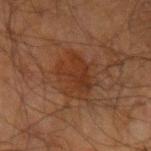<lesion>
<biopsy_status>not biopsied; imaged during a skin examination</biopsy_status>
<automated_metrics>
  <eccentricity>0.65</eccentricity>
  <shape_asymmetry>0.3</shape_asymmetry>
  <cielab_L>28</cielab_L>
  <cielab_a>19</cielab_a>
  <cielab_b>27</cielab_b>
  <vs_skin_darker_L>6.0</vs_skin_darker_L>
  <vs_skin_contrast_norm>6.5</vs_skin_contrast_norm>
  <border_irregularity_0_10>4.0</border_irregularity_0_10>
  <peripheral_color_asymmetry>1.0</peripheral_color_asymmetry>
</automated_metrics>
<site>arm</site>
<lighting>cross-polarized</lighting>
<patient>
  <sex>male</sex>
  <age_approx>70</age_approx>
</patient>
<image>
  <source>total-body photography crop</source>
  <field_of_view_mm>15</field_of_view_mm>
</image>
<lesion_size>
  <long_diameter_mm_approx>5.0</long_diameter_mm_approx>
</lesion_size>
</lesion>